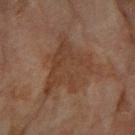<record>
  <biopsy_status>not biopsied; imaged during a skin examination</biopsy_status>
  <patient>
    <sex>female</sex>
    <age_approx>80</age_approx>
  </patient>
  <image>
    <source>total-body photography crop</source>
    <field_of_view_mm>15</field_of_view_mm>
  </image>
  <site>arm</site>
  <lesion_size>
    <long_diameter_mm_approx>7.5</long_diameter_mm_approx>
  </lesion_size>
  <lighting>cross-polarized</lighting>
</record>